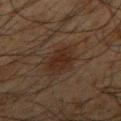Impression:
The lesion was tiled from a total-body skin photograph and was not biopsied.
Acquisition and patient details:
A male patient, in their mid-30s. On the right upper arm. A region of skin cropped from a whole-body photographic capture, roughly 15 mm wide.